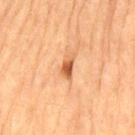Context: Imaged with cross-polarized lighting. The patient is a male aged approximately 85 or older. Measured at roughly 2.5 mm in maximum diameter. The lesion is on the back. The total-body-photography lesion software estimated a within-lesion color-variation index near 2.5/10. A roughly 15 mm field-of-view crop from a total-body skin photograph.